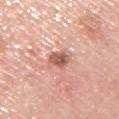subject: male, approximately 60 years of age
lighting: white-light illumination
body site: the back
image: ~15 mm tile from a whole-body skin photo